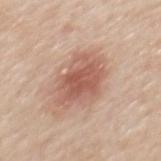Assessment: Recorded during total-body skin imaging; not selected for excision or biopsy.